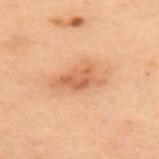Case summary:
- notes · total-body-photography surveillance lesion; no biopsy
- subject · female, roughly 55 years of age
- lighting · cross-polarized
- imaging modality · 15 mm crop, total-body photography
- anatomic site · the upper back